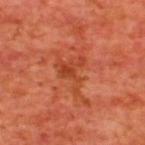The lesion was tiled from a total-body skin photograph and was not biopsied. A close-up tile cropped from a whole-body skin photograph, about 15 mm across. Imaged with cross-polarized lighting. The lesion is on the upper back. The subject is a male in their mid- to late 60s.